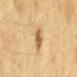<lesion>
  <biopsy_status>not biopsied; imaged during a skin examination</biopsy_status>
  <lighting>cross-polarized</lighting>
  <lesion_size>
    <long_diameter_mm_approx>2.5</long_diameter_mm_approx>
  </lesion_size>
  <image>
    <source>total-body photography crop</source>
    <field_of_view_mm>15</field_of_view_mm>
  </image>
  <patient>
    <sex>female</sex>
    <age_approx>55</age_approx>
  </patient>
  <site>back</site>
  <automated_metrics>
    <cielab_L>53</cielab_L>
    <cielab_a>15</cielab_a>
    <cielab_b>35</cielab_b>
    <vs_skin_darker_L>11.0</vs_skin_darker_L>
    <vs_skin_contrast_norm>7.5</vs_skin_contrast_norm>
    <border_irregularity_0_10>2.5</border_irregularity_0_10>
    <peripheral_color_asymmetry>1.0</peripheral_color_asymmetry>
  </automated_metrics>
</lesion>